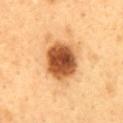follow-up=catalogued during a skin exam; not biopsied | acquisition=total-body-photography crop, ~15 mm field of view | patient=male, aged approximately 50 | body site=the back.